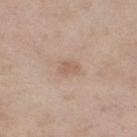Assessment:
Part of a total-body skin-imaging series; this lesion was reviewed on a skin check and was not flagged for biopsy.
Background:
The tile uses white-light illumination. The subject is a female in their mid-50s. The lesion-visualizer software estimated an area of roughly 5 mm², an eccentricity of roughly 0.7, and a shape-asymmetry score of about 0.2 (0 = symmetric). It also reported an average lesion color of about L≈60 a*≈17 b*≈27 (CIELAB) and a lesion-to-skin contrast of about 5 (normalized; higher = more distinct). From the right thigh. The lesion's longest dimension is about 3 mm. A lesion tile, about 15 mm wide, cut from a 3D total-body photograph.